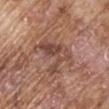Background:
Located on the right upper arm. A 15 mm close-up tile from a total-body photography series done for melanoma screening. Captured under white-light illumination. A male patient aged 73–77.